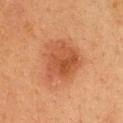workup: no biopsy performed (imaged during a skin exam).